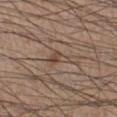A roughly 15 mm field-of-view crop from a total-body skin photograph.
Measured at roughly 3 mm in maximum diameter.
The lesion is on the left lower leg.
Captured under white-light illumination.
A male subject, aged 33–37.